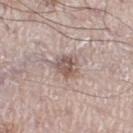Notes:
* workup — imaged on a skin check; not biopsied
* location — the leg
* size — ~3 mm (longest diameter)
* illumination — white-light
* imaging modality — ~15 mm crop, total-body skin-cancer survey
* subject — male, aged 68–72